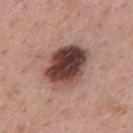notes=total-body-photography surveillance lesion; no biopsy
patient=male, about 40 years old
image source=~15 mm tile from a whole-body skin photo
lighting=white-light illumination
site=the mid back
lesion size=≈6 mm